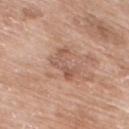Captured during whole-body skin photography for melanoma surveillance; the lesion was not biopsied. A female subject, aged around 70. Located on the chest. A region of skin cropped from a whole-body photographic capture, roughly 15 mm wide. About 4 mm across.